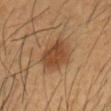The lesion was photographed on a routine skin check and not biopsied; there is no pathology result.
Cropped from a whole-body photographic skin survey; the tile spans about 15 mm.
The lesion's longest dimension is about 4 mm.
The patient is a male in their 40s.
From the head or neck.
The total-body-photography lesion software estimated a mean CIELAB color near L≈40 a*≈18 b*≈31, about 9 CIELAB-L* units darker than the surrounding skin, and a normalized lesion–skin contrast near 8. And it measured a border-irregularity rating of about 2.5/10 and radial color variation of about 1.